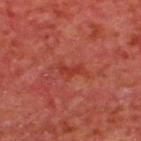The lesion was photographed on a routine skin check and not biopsied; there is no pathology result.
The total-body-photography lesion software estimated an area of roughly 2.5 mm², an eccentricity of roughly 0.9, and two-axis asymmetry of about 0.4. The analysis additionally found border irregularity of about 4 on a 0–10 scale, a within-lesion color-variation index near 0/10, and a peripheral color-asymmetry measure near 0.
Imaged with cross-polarized lighting.
The subject is a male aged 63 to 67.
The lesion is on the upper back.
About 2.5 mm across.
This image is a 15 mm lesion crop taken from a total-body photograph.